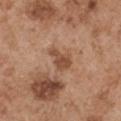The lesion was photographed on a routine skin check and not biopsied; there is no pathology result.
A region of skin cropped from a whole-body photographic capture, roughly 15 mm wide.
Located on the left upper arm.
The subject is a male aged approximately 55.